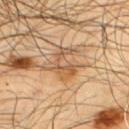Clinical impression: This lesion was catalogued during total-body skin photography and was not selected for biopsy. Background: On the upper back. The patient is a male aged around 65. A close-up tile cropped from a whole-body skin photograph, about 15 mm across.